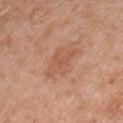Q: Was this lesion biopsied?
A: total-body-photography surveillance lesion; no biopsy
Q: Automated lesion metrics?
A: an area of roughly 10 mm² and a symmetry-axis asymmetry near 0.35; an average lesion color of about L≈56 a*≈23 b*≈33 (CIELAB) and about 7 CIELAB-L* units darker than the surrounding skin; a border-irregularity rating of about 4/10, a color-variation rating of about 3/10, and peripheral color asymmetry of about 1; a classifier nevus-likeness of about 5/100 and lesion-presence confidence of about 100/100
Q: Who is the patient?
A: male, approximately 65 years of age
Q: What lighting was used for the tile?
A: white-light
Q: What is the anatomic site?
A: the front of the torso
Q: What kind of image is this?
A: total-body-photography crop, ~15 mm field of view